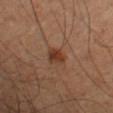notes: imaged on a skin check; not biopsied | acquisition: ~15 mm crop, total-body skin-cancer survey | diameter: ≈2.5 mm | lighting: cross-polarized illumination | site: the arm | subject: male, roughly 65 years of age | TBP lesion metrics: an average lesion color of about L≈36 a*≈20 b*≈29 (CIELAB), about 9 CIELAB-L* units darker than the surrounding skin, and a lesion-to-skin contrast of about 8.5 (normalized; higher = more distinct); a border-irregularity rating of about 1.5/10, a within-lesion color-variation index near 3/10, and peripheral color asymmetry of about 1; a nevus-likeness score of about 95/100 and a lesion-detection confidence of about 100/100.